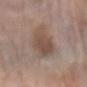Cropped from a total-body skin-imaging series; the visible field is about 15 mm.
An algorithmic analysis of the crop reported a lesion area of about 15 mm², a shape eccentricity near 0.75, and a shape-asymmetry score of about 0.2 (0 = symmetric). The software also gave an average lesion color of about L≈49 a*≈16 b*≈24 (CIELAB), a lesion–skin lightness drop of about 9, and a lesion-to-skin contrast of about 7 (normalized; higher = more distinct). And it measured a nevus-likeness score of about 40/100.
A female subject aged 68 to 72.
From the left forearm.